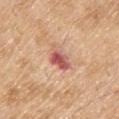image — ~15 mm crop, total-body skin-cancer survey
lighting — white-light
lesion size — about 3.5 mm
subject — male, aged 68–72
anatomic site — the back